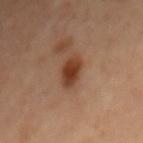lesion_size:
  long_diameter_mm_approx: 4.0
site: mid back
patient:
  sex: female
  age_approx: 65
image:
  source: total-body photography crop
  field_of_view_mm: 15
automated_metrics:
  cielab_L: 38
  cielab_a: 22
  cielab_b: 31
  vs_skin_darker_L: 13.0
  border_irregularity_0_10: 1.5
  color_variation_0_10: 3.5
  peripheral_color_asymmetry: 1.0
  nevus_likeness_0_100: 100
  lesion_detection_confidence_0_100: 100
lighting: cross-polarized